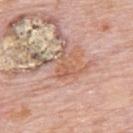{"biopsy_status": "not biopsied; imaged during a skin examination", "site": "back", "automated_metrics": {"area_mm2_approx": 6.5, "eccentricity": 0.6, "shape_asymmetry": 0.6, "cielab_L": 60, "cielab_a": 22, "cielab_b": 31, "vs_skin_darker_L": 8.0, "vs_skin_contrast_norm": 6.0, "border_irregularity_0_10": 8.0, "color_variation_0_10": 3.0, "peripheral_color_asymmetry": 1.0, "nevus_likeness_0_100": 0, "lesion_detection_confidence_0_100": 95}, "image": {"source": "total-body photography crop", "field_of_view_mm": 15}, "lighting": "white-light", "patient": {"sex": "male", "age_approx": 80}, "lesion_size": {"long_diameter_mm_approx": 4.0}}